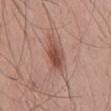biopsy_status: not biopsied; imaged during a skin examination
image:
  source: total-body photography crop
  field_of_view_mm: 15
patient:
  sex: male
  age_approx: 50
site: mid back
lighting: white-light
lesion_size:
  long_diameter_mm_approx: 4.0
automated_metrics:
  area_mm2_approx: 7.5
  eccentricity: 0.8
  shape_asymmetry: 0.15
  border_irregularity_0_10: 2.0
  color_variation_0_10: 4.0
  peripheral_color_asymmetry: 1.0